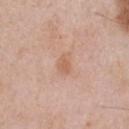notes = imaged on a skin check; not biopsied
lesion size = about 2.5 mm
anatomic site = the chest
TBP lesion metrics = a border-irregularity index near 2/10 and radial color variation of about 0.5; a nevus-likeness score of about 0/100 and a detector confidence of about 100 out of 100 that the crop contains a lesion
acquisition = total-body-photography crop, ~15 mm field of view
subject = male, aged 58–62